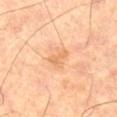workup = imaged on a skin check; not biopsied | acquisition = ~15 mm crop, total-body skin-cancer survey | lighting = cross-polarized illumination | anatomic site = the left thigh | subject = male, about 65 years old.